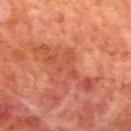Notes:
- notes: no biopsy performed (imaged during a skin exam)
- subject: male, aged 68–72
- automated metrics: a footprint of about 16 mm² and a symmetry-axis asymmetry near 0.4; a border-irregularity index near 8/10, a color-variation rating of about 6/10, and a peripheral color-asymmetry measure near 1.5
- lesion diameter: ~8.5 mm (longest diameter)
- site: the mid back
- lighting: cross-polarized
- imaging modality: ~15 mm crop, total-body skin-cancer survey